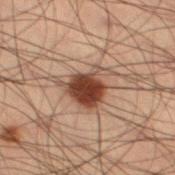follow-up: no biopsy performed (imaged during a skin exam); image source: 15 mm crop, total-body photography; site: the leg; subject: male, approximately 55 years of age; illumination: cross-polarized; lesion diameter: about 5 mm.